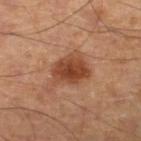The lesion was photographed on a routine skin check and not biopsied; there is no pathology result.